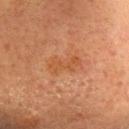Captured during whole-body skin photography for melanoma surveillance; the lesion was not biopsied.
Located on the head or neck.
The tile uses cross-polarized illumination.
The subject is a female roughly 55 years of age.
A 15 mm close-up extracted from a 3D total-body photography capture.